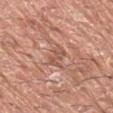This lesion was catalogued during total-body skin photography and was not selected for biopsy. An algorithmic analysis of the crop reported a lesion area of about 4 mm², an eccentricity of roughly 0.6, and a symmetry-axis asymmetry near 0.35. The analysis additionally found about 8 CIELAB-L* units darker than the surrounding skin and a normalized border contrast of about 5.5. The analysis additionally found a classifier nevus-likeness of about 0/100. The lesion is located on the right upper arm. The patient is a male aged approximately 40. The tile uses white-light illumination. A region of skin cropped from a whole-body photographic capture, roughly 15 mm wide.